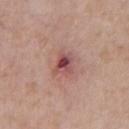| feature | finding |
|---|---|
| biopsy status | imaged on a skin check; not biopsied |
| imaging modality | ~15 mm tile from a whole-body skin photo |
| site | the chest |
| subject | male, approximately 65 years of age |